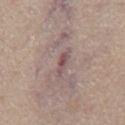Q: Is there a histopathology result?
A: catalogued during a skin exam; not biopsied
Q: Lesion size?
A: ~2.5 mm (longest diameter)
Q: Automated lesion metrics?
A: a lesion area of about 3 mm², an eccentricity of roughly 0.9, and a shape-asymmetry score of about 0.25 (0 = symmetric); a mean CIELAB color near L≈50 a*≈20 b*≈17 and a normalized border contrast of about 7.5; a nevus-likeness score of about 0/100
Q: What kind of image is this?
A: total-body-photography crop, ~15 mm field of view
Q: What is the anatomic site?
A: the mid back
Q: What lighting was used for the tile?
A: white-light
Q: What are the patient's age and sex?
A: male, approximately 70 years of age